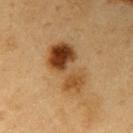workup=no biopsy performed (imaged during a skin exam)
diameter=≈6.5 mm
TBP lesion metrics=a color-variation rating of about 10/10 and a peripheral color-asymmetry measure near 6
site=the right upper arm
image=~15 mm crop, total-body skin-cancer survey
illumination=cross-polarized illumination
patient=male, about 60 years old